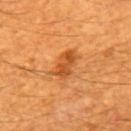| field | value |
|---|---|
| notes | total-body-photography surveillance lesion; no biopsy |
| tile lighting | cross-polarized |
| site | the mid back |
| patient | male, roughly 60 years of age |
| image source | 15 mm crop, total-body photography |